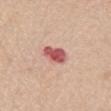| field | value |
|---|---|
| anatomic site | the chest |
| image | ~15 mm tile from a whole-body skin photo |
| patient | male, aged 63–67 |
| automated lesion analysis | a lesion–skin lightness drop of about 16 and a normalized border contrast of about 10.5; a border-irregularity index near 2.5/10 and internal color variation of about 4 on a 0–10 scale; a nevus-likeness score of about 0/100 |